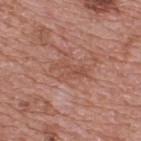workup = no biopsy performed (imaged during a skin exam) | location = the upper back | patient = male, aged approximately 70 | diameter = about 4 mm | lighting = white-light | image = ~15 mm tile from a whole-body skin photo.